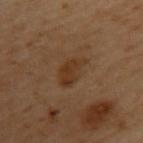Assessment: No biopsy was performed on this lesion — it was imaged during a full skin examination and was not determined to be concerning. Image and clinical context: Captured under cross-polarized illumination. A region of skin cropped from a whole-body photographic capture, roughly 15 mm wide. About 4 mm across. A female subject aged 58 to 62. From the back.